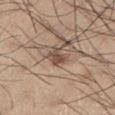Clinical summary: The lesion is on the leg. The patient is a male about 55 years old. Cropped from a total-body skin-imaging series; the visible field is about 15 mm.